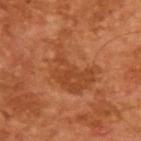follow-up — imaged on a skin check; not biopsied
TBP lesion metrics — an average lesion color of about L≈45 a*≈27 b*≈38 (CIELAB), about 7 CIELAB-L* units darker than the surrounding skin, and a lesion-to-skin contrast of about 5.5 (normalized; higher = more distinct)
subject — male, aged around 65
image source — 15 mm crop, total-body photography
diameter — ≈7.5 mm
illumination — cross-polarized